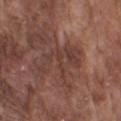Recorded during total-body skin imaging; not selected for excision or biopsy.
Longest diameter approximately 6.5 mm.
Cropped from a total-body skin-imaging series; the visible field is about 15 mm.
On the arm.
Captured under white-light illumination.
A male patient about 75 years old.